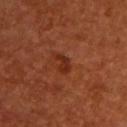Recorded during total-body skin imaging; not selected for excision or biopsy. A 15 mm crop from a total-body photograph taken for skin-cancer surveillance. The patient is a male approximately 55 years of age. Imaged with cross-polarized lighting. Located on the back. The lesion's longest dimension is about 3 mm.